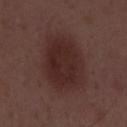Clinical impression: Part of a total-body skin-imaging series; this lesion was reviewed on a skin check and was not flagged for biopsy. Acquisition and patient details: The lesion's longest dimension is about 7.5 mm. Captured under white-light illumination. A male patient aged around 50. A 15 mm crop from a total-body photograph taken for skin-cancer surveillance.